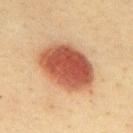<case>
<lighting>cross-polarized</lighting>
<image>
  <source>total-body photography crop</source>
  <field_of_view_mm>15</field_of_view_mm>
</image>
<patient>
  <sex>male</sex>
  <age_approx>40</age_approx>
</patient>
<site>upper back</site>
<lesion_size>
  <long_diameter_mm_approx>7.0</long_diameter_mm_approx>
</lesion_size>
<automated_metrics>
  <eccentricity>0.75</eccentricity>
  <cielab_L>49</cielab_L>
  <cielab_a>26</cielab_a>
  <cielab_b>31</cielab_b>
  <vs_skin_darker_L>17.0</vs_skin_darker_L>
  <vs_skin_contrast_norm>11.5</vs_skin_contrast_norm>
  <border_irregularity_0_10>1.5</border_irregularity_0_10>
  <color_variation_0_10>6.0</color_variation_0_10>
  <peripheral_color_asymmetry>2.0</peripheral_color_asymmetry>
</automated_metrics>
</case>